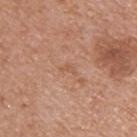This lesion was catalogued during total-body skin photography and was not selected for biopsy. A 15 mm close-up tile from a total-body photography series done for melanoma screening. On the upper back. The patient is a male aged 53 to 57.